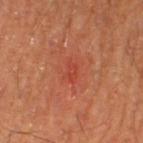Clinical impression:
This lesion was catalogued during total-body skin photography and was not selected for biopsy.